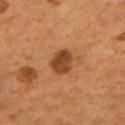Part of a total-body skin-imaging series; this lesion was reviewed on a skin check and was not flagged for biopsy.
Captured under cross-polarized illumination.
The lesion's longest dimension is about 3.5 mm.
The total-body-photography lesion software estimated internal color variation of about 3 on a 0–10 scale and peripheral color asymmetry of about 1.
A male patient, aged around 55.
On the back.
A roughly 15 mm field-of-view crop from a total-body skin photograph.